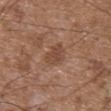This lesion was catalogued during total-body skin photography and was not selected for biopsy. Located on the front of the torso. A male subject, aged around 75. A close-up tile cropped from a whole-body skin photograph, about 15 mm across.